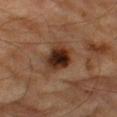The lesion was tiled from a total-body skin photograph and was not biopsied.
On the left thigh.
A close-up tile cropped from a whole-body skin photograph, about 15 mm across.
The patient is a male aged approximately 85 or older.